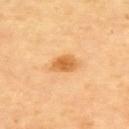Context: A close-up tile cropped from a whole-body skin photograph, about 15 mm across. Longest diameter approximately 3.5 mm. The lesion is located on the upper back. The total-body-photography lesion software estimated a lesion area of about 6 mm² and a shape-asymmetry score of about 0.3 (0 = symmetric). The analysis additionally found border irregularity of about 2.5 on a 0–10 scale and a peripheral color-asymmetry measure near 1.